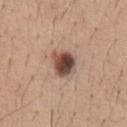follow-up: total-body-photography surveillance lesion; no biopsy | illumination: white-light illumination | automated metrics: an outline eccentricity of about 0.2 (0 = round, 1 = elongated); a border-irregularity rating of about 2/10 and internal color variation of about 9.5 on a 0–10 scale | body site: the abdomen | patient: male, approximately 40 years of age | acquisition: ~15 mm crop, total-body skin-cancer survey | lesion diameter: ≈3.5 mm.